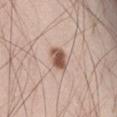A male patient, about 30 years old.
Located on the leg.
Approximately 2.5 mm at its widest.
The total-body-photography lesion software estimated a lesion area of about 5.5 mm², an outline eccentricity of about 0.6 (0 = round, 1 = elongated), and a shape-asymmetry score of about 0.2 (0 = symmetric). It also reported roughly 16 lightness units darker than nearby skin and a normalized border contrast of about 10.5.
A close-up tile cropped from a whole-body skin photograph, about 15 mm across.